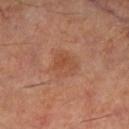Imaged during a routine full-body skin examination; the lesion was not biopsied and no histopathology is available. This is a cross-polarized tile. A roughly 15 mm field-of-view crop from a total-body skin photograph. A male patient, about 60 years old. Measured at roughly 3.5 mm in maximum diameter. Located on the left lower leg.